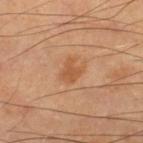{"lesion_size": {"long_diameter_mm_approx": 3.0}, "lighting": "cross-polarized", "automated_metrics": {"cielab_L": 53, "cielab_a": 23, "cielab_b": 36, "vs_skin_darker_L": 7.0, "vs_skin_contrast_norm": 6.0}, "image": {"source": "total-body photography crop", "field_of_view_mm": 15}, "patient": {"sex": "male", "age_approx": 70}, "site": "right lower leg"}